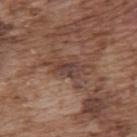– workup · no biopsy performed (imaged during a skin exam)
– image source · ~15 mm crop, total-body skin-cancer survey
– diameter · about 4 mm
– anatomic site · the upper back
– subject · male, aged 73 to 77
– TBP lesion metrics · a lesion area of about 5.5 mm², an outline eccentricity of about 0.85 (0 = round, 1 = elongated), and two-axis asymmetry of about 0.25; an automated nevus-likeness rating near 0 out of 100 and a lesion-detection confidence of about 50/100